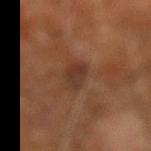workup = catalogued during a skin exam; not biopsied | imaging modality = 15 mm crop, total-body photography | diameter = ~3.5 mm (longest diameter) | location = the right leg | subject = male, in their 60s | automated metrics = a lesion color around L≈33 a*≈19 b*≈27 in CIELAB, roughly 7 lightness units darker than nearby skin, and a normalized lesion–skin contrast near 7.